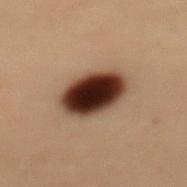workup — imaged on a skin check; not biopsied | diameter — ~5 mm (longest diameter) | automated lesion analysis — a shape eccentricity near 0.7 and two-axis asymmetry of about 0.1; lesion-presence confidence of about 100/100 | acquisition — 15 mm crop, total-body photography | anatomic site — the mid back | tile lighting — cross-polarized illumination | patient — female, aged approximately 50.